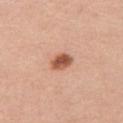{"biopsy_status": "not biopsied; imaged during a skin examination"}